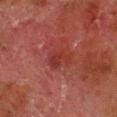Clinical impression:
The lesion was photographed on a routine skin check and not biopsied; there is no pathology result.
Context:
A region of skin cropped from a whole-body photographic capture, roughly 15 mm wide. Automated tile analysis of the lesion measured an area of roughly 5 mm² and an eccentricity of roughly 0.75. And it measured a mean CIELAB color near L≈28 a*≈27 b*≈24, about 5 CIELAB-L* units darker than the surrounding skin, and a normalized lesion–skin contrast near 6. The analysis additionally found a border-irregularity rating of about 3/10, internal color variation of about 2.5 on a 0–10 scale, and peripheral color asymmetry of about 1. The tile uses cross-polarized illumination. The lesion's longest dimension is about 3 mm. A male patient, aged 78–82. The lesion is on the right lower leg.